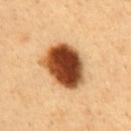No biopsy was performed on this lesion — it was imaged during a full skin examination and was not determined to be concerning. Located on the abdomen. The tile uses cross-polarized illumination. A roughly 15 mm field-of-view crop from a total-body skin photograph. A male subject roughly 50 years of age. The lesion-visualizer software estimated a lesion area of about 21 mm², an outline eccentricity of about 0.65 (0 = round, 1 = elongated), and two-axis asymmetry of about 0.15. The analysis additionally found a mean CIELAB color near L≈47 a*≈26 b*≈39 and a normalized border contrast of about 17. The analysis additionally found a nevus-likeness score of about 100/100 and a detector confidence of about 100 out of 100 that the crop contains a lesion.